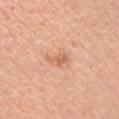workup — total-body-photography surveillance lesion; no biopsy | anatomic site — the chest | lighting — white-light illumination | subject — female, aged around 45 | image — ~15 mm tile from a whole-body skin photo | lesion size — about 2.5 mm.